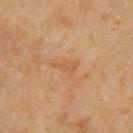Impression:
No biopsy was performed on this lesion — it was imaged during a full skin examination and was not determined to be concerning.
Background:
A female subject about 70 years old. The lesion-visualizer software estimated a lesion color around L≈49 a*≈18 b*≈34 in CIELAB, a lesion–skin lightness drop of about 5, and a normalized lesion–skin contrast near 5. The software also gave a border-irregularity rating of about 5.5/10, a within-lesion color-variation index near 0/10, and a peripheral color-asymmetry measure near 0. The software also gave a classifier nevus-likeness of about 0/100 and lesion-presence confidence of about 100/100. Captured under cross-polarized illumination. A close-up tile cropped from a whole-body skin photograph, about 15 mm across. Measured at roughly 3 mm in maximum diameter. The lesion is located on the left upper arm.